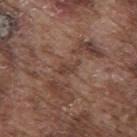follow-up: total-body-photography surveillance lesion; no biopsy | size: about 2.5 mm | imaging modality: ~15 mm tile from a whole-body skin photo | body site: the mid back | subject: male, about 75 years old.